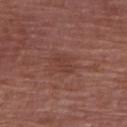The lesion was photographed on a routine skin check and not biopsied; there is no pathology result. A female patient aged 68–72. The lesion's longest dimension is about 3 mm. From the right upper arm. A 15 mm crop from a total-body photograph taken for skin-cancer surveillance. Imaged with white-light lighting.